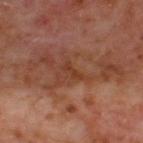A 15 mm crop from a total-body photograph taken for skin-cancer surveillance.
Longest diameter approximately 2.5 mm.
A male subject, aged approximately 60.
From the upper back.